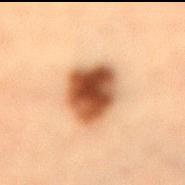Recorded during total-body skin imaging; not selected for excision or biopsy. Longest diameter approximately 5 mm. A 15 mm close-up tile from a total-body photography series done for melanoma screening. An algorithmic analysis of the crop reported about 19 CIELAB-L* units darker than the surrounding skin and a lesion-to-skin contrast of about 13 (normalized; higher = more distinct). The software also gave border irregularity of about 1.5 on a 0–10 scale and internal color variation of about 9 on a 0–10 scale. Located on the lower back. A female subject, aged approximately 40.